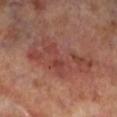notes: catalogued during a skin exam; not biopsied | body site: the left lower leg | tile lighting: cross-polarized | patient: male, aged approximately 70 | TBP lesion metrics: roughly 7 lightness units darker than nearby skin and a lesion-to-skin contrast of about 6 (normalized; higher = more distinct) | diameter: ≈8.5 mm | acquisition: ~15 mm tile from a whole-body skin photo.